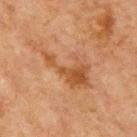workup: imaged on a skin check; not biopsied | image source: total-body-photography crop, ~15 mm field of view | lesion size: ≈8.5 mm | subject: male, about 65 years old | TBP lesion metrics: a lesion color around L≈43 a*≈20 b*≈32 in CIELAB and a lesion–skin lightness drop of about 7; a border-irregularity rating of about 9.5/10, internal color variation of about 4.5 on a 0–10 scale, and a peripheral color-asymmetry measure near 1.5; a detector confidence of about 100 out of 100 that the crop contains a lesion | location: the chest.